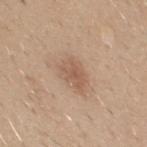| key | value |
|---|---|
| follow-up | total-body-photography surveillance lesion; no biopsy |
| subject | male, aged around 40 |
| body site | the mid back |
| lesion diameter | ≈4 mm |
| image | total-body-photography crop, ~15 mm field of view |
| lighting | white-light |